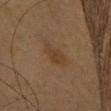This lesion was catalogued during total-body skin photography and was not selected for biopsy.
Longest diameter approximately 3.5 mm.
A 15 mm close-up tile from a total-body photography series done for melanoma screening.
The patient is a female roughly 60 years of age.
The tile uses cross-polarized illumination.
The lesion is located on the head or neck.
Automated image analysis of the tile measured a lesion area of about 5.5 mm² and two-axis asymmetry of about 0.3. It also reported about 5 CIELAB-L* units darker than the surrounding skin. And it measured border irregularity of about 3.5 on a 0–10 scale, a color-variation rating of about 2/10, and peripheral color asymmetry of about 0.5. The software also gave an automated nevus-likeness rating near 15 out of 100 and a lesion-detection confidence of about 100/100.